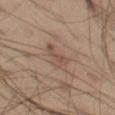This lesion was catalogued during total-body skin photography and was not selected for biopsy. Located on the left thigh. A 15 mm crop from a total-body photograph taken for skin-cancer surveillance. The tile uses cross-polarized illumination. The patient is a male in their 40s. The lesion's longest dimension is about 3.5 mm.